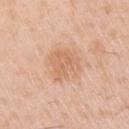This lesion was catalogued during total-body skin photography and was not selected for biopsy. This is a white-light tile. A male patient about 50 years old. About 4 mm across. The lesion is on the right upper arm. Cropped from a whole-body photographic skin survey; the tile spans about 15 mm.